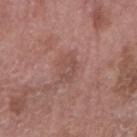Case summary:
* site · the right forearm
* imaging modality · ~15 mm crop, total-body skin-cancer survey
* subject · male, aged around 75
* lighting · white-light
* diameter · about 3 mm